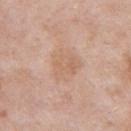Captured during whole-body skin photography for melanoma surveillance; the lesion was not biopsied.
Approximately 3.5 mm at its widest.
A 15 mm close-up tile from a total-body photography series done for melanoma screening.
The subject is a male aged approximately 55.
Located on the left upper arm.